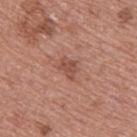This lesion was catalogued during total-body skin photography and was not selected for biopsy. A 15 mm close-up tile from a total-body photography series done for melanoma screening. On the upper back. The lesion's longest dimension is about 2.5 mm. The subject is a male about 55 years old. Automated tile analysis of the lesion measured about 9 CIELAB-L* units darker than the surrounding skin and a lesion-to-skin contrast of about 6.5 (normalized; higher = more distinct). The software also gave border irregularity of about 4 on a 0–10 scale and radial color variation of about 0.5.